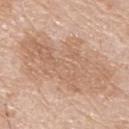Q: What kind of image is this?
A: ~15 mm crop, total-body skin-cancer survey
Q: What is the lesion's diameter?
A: about 11 mm
Q: Automated lesion metrics?
A: a shape-asymmetry score of about 0.3 (0 = symmetric); border irregularity of about 5.5 on a 0–10 scale, internal color variation of about 4 on a 0–10 scale, and peripheral color asymmetry of about 1.5; an automated nevus-likeness rating near 0 out of 100
Q: Where on the body is the lesion?
A: the upper back
Q: What are the patient's age and sex?
A: male, aged 78 to 82